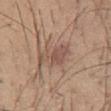Impression:
No biopsy was performed on this lesion — it was imaged during a full skin examination and was not determined to be concerning.
Acquisition and patient details:
A 15 mm close-up tile from a total-body photography series done for melanoma screening. Captured under white-light illumination. From the chest. Approximately 4 mm at its widest. An algorithmic analysis of the crop reported a lesion area of about 7.5 mm², a shape eccentricity near 0.7, and two-axis asymmetry of about 0.55. The analysis additionally found an average lesion color of about L≈52 a*≈17 b*≈26 (CIELAB), about 8 CIELAB-L* units darker than the surrounding skin, and a normalized lesion–skin contrast near 6. The software also gave border irregularity of about 6.5 on a 0–10 scale, a color-variation rating of about 2.5/10, and a peripheral color-asymmetry measure near 1. The analysis additionally found a classifier nevus-likeness of about 0/100 and a lesion-detection confidence of about 100/100. The subject is a male aged 33 to 37.